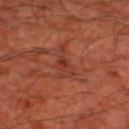Notes:
* workup · total-body-photography surveillance lesion; no biopsy
* automated metrics · a lesion area of about 5 mm² and a symmetry-axis asymmetry near 0.55; a lesion color around L≈37 a*≈29 b*≈31 in CIELAB and roughly 6 lightness units darker than nearby skin
* lesion diameter · ≈4 mm
* body site · the left thigh
* image source · 15 mm crop, total-body photography
* subject · in their mid- to late 60s
* tile lighting · cross-polarized illumination